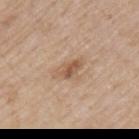biopsy status: catalogued during a skin exam; not biopsied
site: the left upper arm
subject: male, aged 73–77
imaging modality: total-body-photography crop, ~15 mm field of view